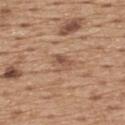Impression:
No biopsy was performed on this lesion — it was imaged during a full skin examination and was not determined to be concerning.
Clinical summary:
Automated image analysis of the tile measured a lesion area of about 4 mm², a shape eccentricity near 0.6, and two-axis asymmetry of about 0.35. It also reported a lesion color around L≈53 a*≈19 b*≈30 in CIELAB, roughly 8 lightness units darker than nearby skin, and a lesion-to-skin contrast of about 6 (normalized; higher = more distinct). The software also gave an automated nevus-likeness rating near 0 out of 100 and a detector confidence of about 100 out of 100 that the crop contains a lesion. A roughly 15 mm field-of-view crop from a total-body skin photograph. Imaged with white-light lighting. Approximately 2.5 mm at its widest. The lesion is on the upper back. The subject is a male in their mid- to late 60s.